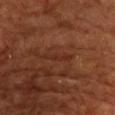Part of a total-body skin-imaging series; this lesion was reviewed on a skin check and was not flagged for biopsy.
The subject is a male in their 60s.
A lesion tile, about 15 mm wide, cut from a 3D total-body photograph.
The lesion is located on the chest.